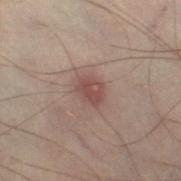Acquisition and patient details:
Cropped from a whole-body photographic skin survey; the tile spans about 15 mm. The lesion-visualizer software estimated a footprint of about 5.5 mm², an eccentricity of roughly 0.65, and a shape-asymmetry score of about 0.2 (0 = symmetric). It also reported a border-irregularity index near 2/10 and a within-lesion color-variation index near 2/10. This is a cross-polarized tile. Approximately 3 mm at its widest. Located on the leg. A male patient, aged 48–52.